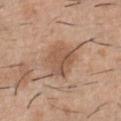Notes:
- image · ~15 mm crop, total-body skin-cancer survey
- size · ≈4 mm
- patient · male, aged 38 to 42
- illumination · white-light illumination
- site · the chest